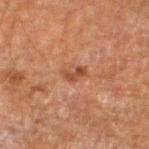* notes: catalogued during a skin exam; not biopsied
* site: the left lower leg
* imaging modality: ~15 mm tile from a whole-body skin photo
* subject: male, aged approximately 60
* tile lighting: cross-polarized